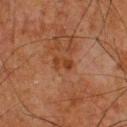No biopsy was performed on this lesion — it was imaged during a full skin examination and was not determined to be concerning.
A male patient aged 63–67.
The lesion is located on the upper back.
Longest diameter approximately 2.5 mm.
Cropped from a total-body skin-imaging series; the visible field is about 15 mm.
The lesion-visualizer software estimated a lesion area of about 3 mm² and an eccentricity of roughly 0.9. It also reported a mean CIELAB color near L≈32 a*≈21 b*≈30, roughly 6 lightness units darker than nearby skin, and a normalized lesion–skin contrast near 7. The analysis additionally found lesion-presence confidence of about 100/100.